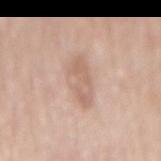Findings:
- notes: total-body-photography surveillance lesion; no biopsy